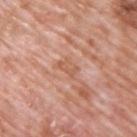Case summary:
• workup · no biopsy performed (imaged during a skin exam)
• location · the upper back
• TBP lesion metrics · an area of roughly 3 mm², an outline eccentricity of about 0.85 (0 = round, 1 = elongated), and a shape-asymmetry score of about 0.35 (0 = symmetric); a mean CIELAB color near L≈58 a*≈24 b*≈32 and a normalized border contrast of about 5.5; a classifier nevus-likeness of about 0/100 and a lesion-detection confidence of about 100/100
• tile lighting · white-light
• lesion diameter · about 3 mm
• image · ~15 mm tile from a whole-body skin photo
• subject · male, aged 68 to 72